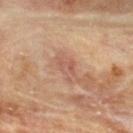Assessment:
Captured during whole-body skin photography for melanoma surveillance; the lesion was not biopsied.
Acquisition and patient details:
A 15 mm close-up extracted from a 3D total-body photography capture. Measured at roughly 3 mm in maximum diameter. The patient is a male aged 83 or older. The tile uses cross-polarized illumination. Automated image analysis of the tile measured an area of roughly 4.5 mm², an outline eccentricity of about 0.8 (0 = round, 1 = elongated), and a symmetry-axis asymmetry near 0.3. The analysis additionally found a border-irregularity index near 3/10 and a within-lesion color-variation index near 2.5/10. The lesion is on the upper back.